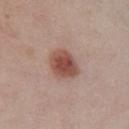{
  "biopsy_status": "not biopsied; imaged during a skin examination",
  "automated_metrics": {
    "nevus_likeness_0_100": 100,
    "lesion_detection_confidence_0_100": 100
  },
  "image": {
    "source": "total-body photography crop",
    "field_of_view_mm": 15
  },
  "patient": {
    "sex": "female",
    "age_approx": 65
  },
  "lighting": "white-light",
  "site": "chest"
}